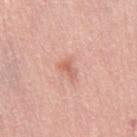Captured during whole-body skin photography for melanoma surveillance; the lesion was not biopsied.
Automated image analysis of the tile measured a classifier nevus-likeness of about 0/100 and lesion-presence confidence of about 100/100.
The tile uses white-light illumination.
A female subject aged approximately 65.
The lesion is on the right thigh.
Cropped from a total-body skin-imaging series; the visible field is about 15 mm.